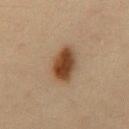location=the chest
patient=male, in their mid-30s
lesion diameter=≈5 mm
image=~15 mm tile from a whole-body skin photo
tile lighting=cross-polarized illumination